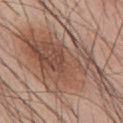Context:
Located on the abdomen. This is a white-light tile. The total-body-photography lesion software estimated a color-variation rating of about 7/10 and radial color variation of about 2.5. Cropped from a whole-body photographic skin survey; the tile spans about 15 mm. The recorded lesion diameter is about 13 mm. The subject is a male aged approximately 60.